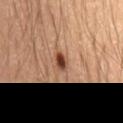Part of a total-body skin-imaging series; this lesion was reviewed on a skin check and was not flagged for biopsy.
Cropped from a total-body skin-imaging series; the visible field is about 15 mm.
On the mid back.
Longest diameter approximately 2.5 mm.
This is a cross-polarized tile.
A male subject roughly 55 years of age.
The lesion-visualizer software estimated a footprint of about 3 mm². And it measured a lesion color around L≈43 a*≈23 b*≈31 in CIELAB, roughly 15 lightness units darker than nearby skin, and a lesion-to-skin contrast of about 11 (normalized; higher = more distinct). And it measured internal color variation of about 3.5 on a 0–10 scale and a peripheral color-asymmetry measure near 0.5.